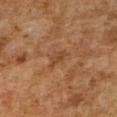Recorded during total-body skin imaging; not selected for excision or biopsy. The tile uses cross-polarized illumination. The total-body-photography lesion software estimated an eccentricity of roughly 0.8 and a shape-asymmetry score of about 0.5 (0 = symmetric). It also reported an average lesion color of about L≈39 a*≈19 b*≈31 (CIELAB), a lesion–skin lightness drop of about 5, and a normalized border contrast of about 4.5. The software also gave a border-irregularity index near 5/10, a within-lesion color-variation index near 2/10, and radial color variation of about 0.5. The software also gave a nevus-likeness score of about 0/100 and a detector confidence of about 100 out of 100 that the crop contains a lesion. Longest diameter approximately 3.5 mm. A female subject aged around 60. A region of skin cropped from a whole-body photographic capture, roughly 15 mm wide. On the right upper arm.